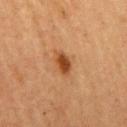Clinical impression: Imaged during a routine full-body skin examination; the lesion was not biopsied and no histopathology is available. Clinical summary: Captured under cross-polarized illumination. From the right upper arm. The patient is a female in their 60s. The lesion-visualizer software estimated a lesion color around L≈41 a*≈24 b*≈37 in CIELAB and a lesion-to-skin contrast of about 10 (normalized; higher = more distinct). The software also gave a within-lesion color-variation index near 2/10 and a peripheral color-asymmetry measure near 0.5. A region of skin cropped from a whole-body photographic capture, roughly 15 mm wide. The lesion's longest dimension is about 3 mm.